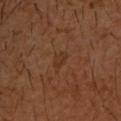Q: Is there a histopathology result?
A: total-body-photography surveillance lesion; no biopsy
Q: What kind of image is this?
A: total-body-photography crop, ~15 mm field of view
Q: Where on the body is the lesion?
A: the upper back
Q: Who is the patient?
A: male, aged 28–32